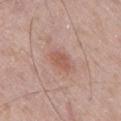Assessment: This lesion was catalogued during total-body skin photography and was not selected for biopsy. Image and clinical context: Automated image analysis of the tile measured a footprint of about 4.5 mm² and an eccentricity of roughly 0.7. The analysis additionally found a mean CIELAB color near L≈56 a*≈22 b*≈27 and a lesion-to-skin contrast of about 6 (normalized; higher = more distinct). A male patient in their 70s. The tile uses white-light illumination. On the right thigh. A lesion tile, about 15 mm wide, cut from a 3D total-body photograph.